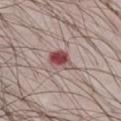– notes · catalogued during a skin exam; not biopsied
– body site · the leg
– lighting · white-light illumination
– patient · male, aged around 40
– imaging modality · ~15 mm tile from a whole-body skin photo